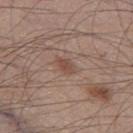Q: Was a biopsy performed?
A: catalogued during a skin exam; not biopsied
Q: What is the lesion's diameter?
A: ~2.5 mm (longest diameter)
Q: What is the imaging modality?
A: total-body-photography crop, ~15 mm field of view
Q: How was the tile lit?
A: white-light
Q: Patient demographics?
A: male, approximately 45 years of age
Q: Lesion location?
A: the left thigh